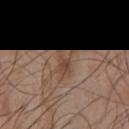Notes:
* tile lighting — white-light illumination
* patient — male, aged 53–57
* imaging modality — total-body-photography crop, ~15 mm field of view
* location — the front of the torso